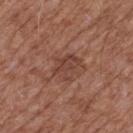| feature | finding |
|---|---|
| anatomic site | the back |
| image source | 15 mm crop, total-body photography |
| diameter | ≈3.5 mm |
| subject | male, about 75 years old |
| automated lesion analysis | a border-irregularity rating of about 5/10 |
| lighting | white-light |